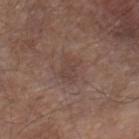Case summary:
* workup: imaged on a skin check; not biopsied
* image: 15 mm crop, total-body photography
* automated metrics: an area of roughly 5.5 mm², a shape eccentricity near 0.8, and a shape-asymmetry score of about 0.25 (0 = symmetric); about 6 CIELAB-L* units darker than the surrounding skin and a lesion-to-skin contrast of about 5 (normalized; higher = more distinct)
* patient: male, about 80 years old
* lighting: white-light
* lesion size: ≈3 mm
* body site: the right lower leg